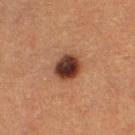A roughly 15 mm field-of-view crop from a total-body skin photograph. From the left lower leg. Imaged with cross-polarized lighting. The total-body-photography lesion software estimated a footprint of about 8.5 mm², an eccentricity of roughly 0.5, and a shape-asymmetry score of about 0.2 (0 = symmetric). It also reported a border-irregularity index near 2/10 and a within-lesion color-variation index near 6.5/10. And it measured a nevus-likeness score of about 95/100. A female subject approximately 40 years of age. About 3.5 mm across.